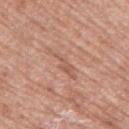Part of a total-body skin-imaging series; this lesion was reviewed on a skin check and was not flagged for biopsy.
Imaged with white-light lighting.
A close-up tile cropped from a whole-body skin photograph, about 15 mm across.
Longest diameter approximately 4.5 mm.
The lesion is on the right upper arm.
A female subject about 60 years old.